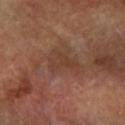image = ~15 mm tile from a whole-body skin photo
anatomic site = the left arm
patient = female, approximately 60 years of age